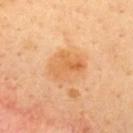Notes:
- notes: catalogued during a skin exam; not biopsied
- lighting: cross-polarized illumination
- image: ~15 mm tile from a whole-body skin photo
- image-analysis metrics: a mean CIELAB color near L≈66 a*≈25 b*≈44 and a lesion-to-skin contrast of about 6.5 (normalized; higher = more distinct); an automated nevus-likeness rating near 10 out of 100
- lesion size: about 4.5 mm
- anatomic site: the upper back
- patient: male, roughly 40 years of age